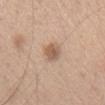Notes:
- notes: total-body-photography surveillance lesion; no biopsy
- anatomic site: the head or neck
- lighting: white-light
- imaging modality: ~15 mm tile from a whole-body skin photo
- subject: female, in their 40s
- size: ≈3 mm